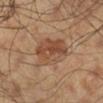Captured during whole-body skin photography for melanoma surveillance; the lesion was not biopsied. A close-up tile cropped from a whole-body skin photograph, about 15 mm across. The patient is a male in their mid-40s. The lesion is located on the right lower leg.